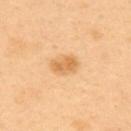biopsy_status: not biopsied; imaged during a skin examination
lighting: cross-polarized
patient:
  sex: male
  age_approx: 40
image:
  source: total-body photography crop
  field_of_view_mm: 15
lesion_size:
  long_diameter_mm_approx: 3.0
site: upper back